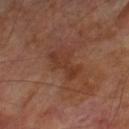biopsy status = total-body-photography surveillance lesion; no biopsy
image-analysis metrics = a footprint of about 6.5 mm² and a symmetry-axis asymmetry near 0.45; a mean CIELAB color near L≈35 a*≈22 b*≈28, a lesion–skin lightness drop of about 6, and a normalized border contrast of about 6
location = the right lower leg
image source = ~15 mm crop, total-body skin-cancer survey
patient = male, roughly 70 years of age
diameter = ≈4.5 mm